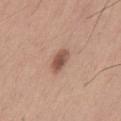Assessment:
No biopsy was performed on this lesion — it was imaged during a full skin examination and was not determined to be concerning.
Acquisition and patient details:
A 15 mm close-up extracted from a 3D total-body photography capture. The lesion is on the mid back. A male patient, aged around 65.